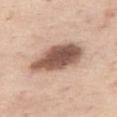Case summary:
- notes · catalogued during a skin exam; not biopsied
- subject · female, aged approximately 55
- lesion diameter · ~7 mm (longest diameter)
- acquisition · ~15 mm crop, total-body skin-cancer survey
- site · the right thigh
- automated lesion analysis · a footprint of about 20 mm² and a symmetry-axis asymmetry near 0.15; an average lesion color of about L≈56 a*≈18 b*≈26 (CIELAB); a nevus-likeness score of about 75/100 and lesion-presence confidence of about 100/100